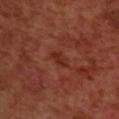Imaged during a routine full-body skin examination; the lesion was not biopsied and no histopathology is available. Cropped from a whole-body photographic skin survey; the tile spans about 15 mm. A male subject about 70 years old. Located on the back. Measured at roughly 2.5 mm in maximum diameter. Captured under cross-polarized illumination.